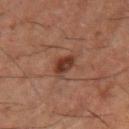The lesion was photographed on a routine skin check and not biopsied; there is no pathology result. On the left upper arm. A 15 mm close-up extracted from a 3D total-body photography capture. Automated tile analysis of the lesion measured a lesion area of about 4.5 mm², an outline eccentricity of about 0.55 (0 = round, 1 = elongated), and a symmetry-axis asymmetry near 0.25. The analysis additionally found an automated nevus-likeness rating near 85 out of 100. A male subject, in their 50s.